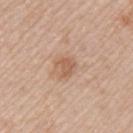No biopsy was performed on this lesion — it was imaged during a full skin examination and was not determined to be concerning.